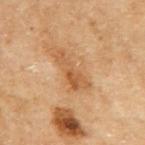| field | value |
|---|---|
| notes | imaged on a skin check; not biopsied |